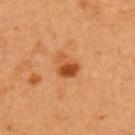Imaged during a routine full-body skin examination; the lesion was not biopsied and no histopathology is available.
Located on the upper back.
Cropped from a total-body skin-imaging series; the visible field is about 15 mm.
The tile uses cross-polarized illumination.
Automated image analysis of the tile measured a lesion area of about 5.5 mm², a shape eccentricity near 0.6, and a shape-asymmetry score of about 0.4 (0 = symmetric). And it measured a within-lesion color-variation index near 3.5/10 and peripheral color asymmetry of about 1.
A female patient aged approximately 40.
Longest diameter approximately 3 mm.